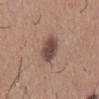Cropped from a total-body skin-imaging series; the visible field is about 15 mm.
This is a white-light tile.
About 5 mm across.
Automated tile analysis of the lesion measured a footprint of about 8 mm², a shape eccentricity near 0.9, and a shape-asymmetry score of about 0.25 (0 = symmetric). And it measured a classifier nevus-likeness of about 80/100 and a detector confidence of about 100 out of 100 that the crop contains a lesion.
From the mid back.
A male patient, roughly 65 years of age.